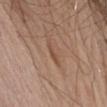Assessment: Captured during whole-body skin photography for melanoma surveillance; the lesion was not biopsied. Acquisition and patient details: The lesion is on the right upper arm. A male patient, approximately 75 years of age. Cropped from a total-body skin-imaging series; the visible field is about 15 mm. The recorded lesion diameter is about 3 mm. Imaged with white-light lighting.